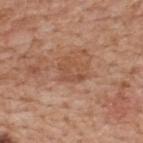– follow-up: total-body-photography surveillance lesion; no biopsy
– tile lighting: white-light illumination
– imaging modality: 15 mm crop, total-body photography
– anatomic site: the upper back
– subject: male, in their mid- to late 60s
– lesion size: about 3 mm
– image-analysis metrics: a nevus-likeness score of about 5/100